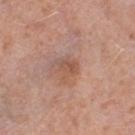Impression: Imaged during a routine full-body skin examination; the lesion was not biopsied and no histopathology is available. Acquisition and patient details: The subject is a female approximately 40 years of age. Located on the left thigh. The lesion-visualizer software estimated a lesion area of about 4.5 mm², an outline eccentricity of about 0.65 (0 = round, 1 = elongated), and a shape-asymmetry score of about 0.3 (0 = symmetric). The analysis additionally found an average lesion color of about L≈54 a*≈21 b*≈28 (CIELAB), about 8 CIELAB-L* units darker than the surrounding skin, and a lesion-to-skin contrast of about 5.5 (normalized; higher = more distinct). The analysis additionally found a detector confidence of about 100 out of 100 that the crop contains a lesion. A 15 mm close-up extracted from a 3D total-body photography capture. Imaged with white-light lighting.